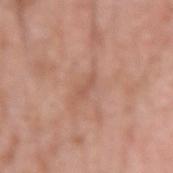Clinical impression: Recorded during total-body skin imaging; not selected for excision or biopsy. Image and clinical context: From the arm. A male subject roughly 60 years of age. A lesion tile, about 15 mm wide, cut from a 3D total-body photograph.